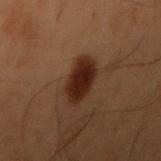Imaged during a routine full-body skin examination; the lesion was not biopsied and no histopathology is available.
A male subject aged 53 to 57.
About 4 mm across.
On the right upper arm.
A roughly 15 mm field-of-view crop from a total-body skin photograph.